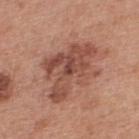  biopsy_status: not biopsied; imaged during a skin examination
  image:
    source: total-body photography crop
    field_of_view_mm: 15
  automated_metrics:
    area_mm2_approx: 24.0
    eccentricity: 0.75
    shape_asymmetry: 0.45
    cielab_L: 49
    cielab_a: 24
    cielab_b: 28
    vs_skin_darker_L: 11.0
    vs_skin_contrast_norm: 7.5
  site: upper back
  patient:
    sex: male
    age_approx: 30
  lighting: white-light
  lesion_size:
    long_diameter_mm_approx: 7.5